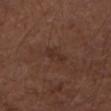The lesion was photographed on a routine skin check and not biopsied; there is no pathology result.
Automated tile analysis of the lesion measured an eccentricity of roughly 0.9 and two-axis asymmetry of about 0.5. It also reported a mean CIELAB color near L≈30 a*≈18 b*≈23, roughly 5 lightness units darker than nearby skin, and a lesion-to-skin contrast of about 6 (normalized; higher = more distinct). It also reported a border-irregularity rating of about 6/10, internal color variation of about 0 on a 0–10 scale, and peripheral color asymmetry of about 0.
The subject is a male aged approximately 75.
From the left forearm.
The recorded lesion diameter is about 2.5 mm.
Captured under white-light illumination.
A roughly 15 mm field-of-view crop from a total-body skin photograph.